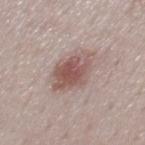Notes:
• biopsy status — imaged on a skin check; not biopsied
• imaging modality — ~15 mm crop, total-body skin-cancer survey
• patient — male, aged 48–52
• lighting — white-light illumination
• body site — the mid back
• diameter — ≈5 mm